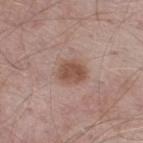Notes:
* follow-up · no biopsy performed (imaged during a skin exam)
* body site · the leg
* lesion size · about 3.5 mm
* automated lesion analysis · a shape eccentricity near 0.6 and two-axis asymmetry of about 0.2; a mean CIELAB color near L≈52 a*≈19 b*≈26 and a normalized lesion–skin contrast near 7.5; an automated nevus-likeness rating near 70 out of 100 and a lesion-detection confidence of about 100/100
* tile lighting · white-light illumination
* subject · male, in their mid- to late 50s
* imaging modality · ~15 mm crop, total-body skin-cancer survey